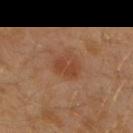<record>
  <biopsy_status>not biopsied; imaged during a skin examination</biopsy_status>
  <patient>
    <sex>male</sex>
    <age_approx>30</age_approx>
  </patient>
  <site>left arm</site>
  <automated_metrics>
    <cielab_L>42</cielab_L>
    <cielab_a>23</cielab_a>
    <cielab_b>32</cielab_b>
    <vs_skin_contrast_norm>6.5</vs_skin_contrast_norm>
    <border_irregularity_0_10>3.0</border_irregularity_0_10>
    <color_variation_0_10>2.5</color_variation_0_10>
    <peripheral_color_asymmetry>1.0</peripheral_color_asymmetry>
    <nevus_likeness_0_100>50</nevus_likeness_0_100>
    <lesion_detection_confidence_0_100>100</lesion_detection_confidence_0_100>
  </automated_metrics>
  <image>
    <source>total-body photography crop</source>
    <field_of_view_mm>15</field_of_view_mm>
  </image>
</record>